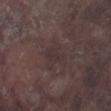• biopsy status — catalogued during a skin exam; not biopsied
• acquisition — total-body-photography crop, ~15 mm field of view
• size — ~3 mm (longest diameter)
• location — the right lower leg
• patient — male, aged 73–77